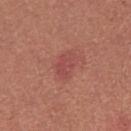No biopsy was performed on this lesion — it was imaged during a full skin examination and was not determined to be concerning. Cropped from a total-body skin-imaging series; the visible field is about 15 mm. Located on the left upper arm. About 3 mm across. The subject is a female about 25 years old.